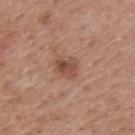Recorded during total-body skin imaging; not selected for excision or biopsy. Cropped from a total-body skin-imaging series; the visible field is about 15 mm. From the mid back. Automated tile analysis of the lesion measured a border-irregularity rating of about 2.5/10, a within-lesion color-variation index near 5/10, and a peripheral color-asymmetry measure near 2. Approximately 3 mm at its widest. The subject is a male aged 53 to 57. The tile uses white-light illumination.